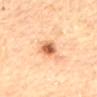This lesion was catalogued during total-body skin photography and was not selected for biopsy. An algorithmic analysis of the crop reported a lesion area of about 5.5 mm², an eccentricity of roughly 0.75, and a symmetry-axis asymmetry near 0.2. The analysis additionally found a border-irregularity rating of about 2/10 and internal color variation of about 7 on a 0–10 scale. And it measured lesion-presence confidence of about 100/100. A 15 mm close-up tile from a total-body photography series done for melanoma screening. The recorded lesion diameter is about 3.5 mm. Captured under cross-polarized illumination. A male subject aged 83 or over. The lesion is located on the back.